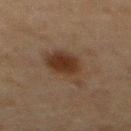{"biopsy_status": "not biopsied; imaged during a skin examination", "patient": {"sex": "male", "age_approx": 75}, "image": {"source": "total-body photography crop", "field_of_view_mm": 15}, "lesion_size": {"long_diameter_mm_approx": 5.5}, "site": "abdomen", "lighting": "cross-polarized"}